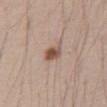follow-up: catalogued during a skin exam; not biopsied
acquisition: ~15 mm tile from a whole-body skin photo
lesion size: about 2.5 mm
patient: male, aged 38–42
TBP lesion metrics: an eccentricity of roughly 0.4 and a symmetry-axis asymmetry near 0.2; an automated nevus-likeness rating near 100 out of 100 and a lesion-detection confidence of about 100/100
illumination: white-light illumination
location: the abdomen